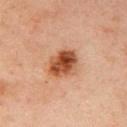Impression: Recorded during total-body skin imaging; not selected for excision or biopsy. Clinical summary: A 15 mm close-up extracted from a 3D total-body photography capture. A male patient, aged 43 to 47. This is a cross-polarized tile. From the right upper arm.